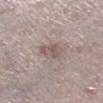This image is a 15 mm lesion crop taken from a total-body photograph. Imaged with white-light lighting. The lesion is located on the right lower leg. A male subject aged 78 to 82. The total-body-photography lesion software estimated a shape eccentricity near 0.75 and a shape-asymmetry score of about 0.2 (0 = symmetric). The recorded lesion diameter is about 3.5 mm.